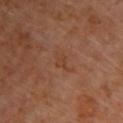Impression:
The lesion was tiled from a total-body skin photograph and was not biopsied.
Clinical summary:
A male patient aged approximately 60. The lesion's longest dimension is about 3 mm. The lesion is on the upper back. A close-up tile cropped from a whole-body skin photograph, about 15 mm across. This is a cross-polarized tile. An algorithmic analysis of the crop reported a shape-asymmetry score of about 0.3 (0 = symmetric). The analysis additionally found a mean CIELAB color near L≈39 a*≈21 b*≈30 and a lesion–skin lightness drop of about 5. The software also gave border irregularity of about 3.5 on a 0–10 scale, a within-lesion color-variation index near 1/10, and a peripheral color-asymmetry measure near 0.5. The analysis additionally found a classifier nevus-likeness of about 0/100 and lesion-presence confidence of about 100/100.